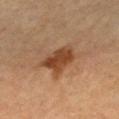Q: Is there a histopathology result?
A: imaged on a skin check; not biopsied
Q: What are the patient's age and sex?
A: female, aged around 60
Q: How was this image acquired?
A: total-body-photography crop, ~15 mm field of view
Q: What is the anatomic site?
A: the right forearm